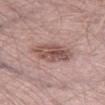notes = total-body-photography surveillance lesion; no biopsy | image source = total-body-photography crop, ~15 mm field of view | subject = male, approximately 50 years of age | illumination = white-light illumination | anatomic site = the leg | image-analysis metrics = a mean CIELAB color near L≈52 a*≈20 b*≈22, roughly 12 lightness units darker than nearby skin, and a normalized border contrast of about 8; a border-irregularity rating of about 2/10, a within-lesion color-variation index near 5/10, and peripheral color asymmetry of about 1.5 | lesion size = about 5 mm.